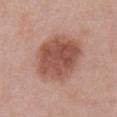| field | value |
|---|---|
| follow-up | no biopsy performed (imaged during a skin exam) |
| diameter | about 6.5 mm |
| site | the front of the torso |
| automated metrics | a lesion color around L≈52 a*≈24 b*≈27 in CIELAB, a lesion–skin lightness drop of about 13, and a normalized border contrast of about 9; border irregularity of about 1.5 on a 0–10 scale and radial color variation of about 1.5 |
| illumination | white-light |
| patient | female, roughly 40 years of age |
| image | 15 mm crop, total-body photography |